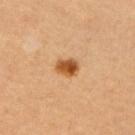Impression: The lesion was photographed on a routine skin check and not biopsied; there is no pathology result. Clinical summary: The total-body-photography lesion software estimated a footprint of about 4.5 mm², an outline eccentricity of about 0.65 (0 = round, 1 = elongated), and a shape-asymmetry score of about 0.2 (0 = symmetric). The software also gave a nevus-likeness score of about 100/100 and lesion-presence confidence of about 100/100. A female patient approximately 50 years of age. A 15 mm close-up tile from a total-body photography series done for melanoma screening.